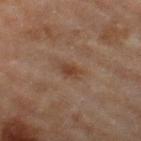{"biopsy_status": "not biopsied; imaged during a skin examination", "image": {"source": "total-body photography crop", "field_of_view_mm": 15}, "patient": {"sex": "female", "age_approx": 60}, "lighting": "cross-polarized", "site": "left thigh", "lesion_size": {"long_diameter_mm_approx": 3.0}, "automated_metrics": {"cielab_L": 37, "cielab_a": 17, "cielab_b": 26, "vs_skin_contrast_norm": 7.0}}